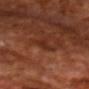Q: Is there a histopathology result?
A: total-body-photography surveillance lesion; no biopsy
Q: Automated lesion metrics?
A: a footprint of about 3 mm², a shape eccentricity near 0.9, and two-axis asymmetry of about 0.35; about 6 CIELAB-L* units darker than the surrounding skin and a normalized border contrast of about 6; a border-irregularity rating of about 3.5/10
Q: Illumination type?
A: cross-polarized illumination
Q: How was this image acquired?
A: ~15 mm crop, total-body skin-cancer survey
Q: What is the lesion's diameter?
A: ≈2.5 mm
Q: Patient demographics?
A: male, aged 68–72
Q: What is the anatomic site?
A: the chest